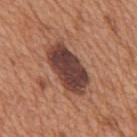This image is a 15 mm lesion crop taken from a total-body photograph.
Captured under white-light illumination.
A male patient, aged approximately 65.
From the mid back.
The lesion's longest dimension is about 6.5 mm.